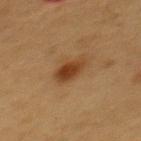illumination = cross-polarized illumination
imaging modality = ~15 mm tile from a whole-body skin photo
diameter = ~3.5 mm (longest diameter)
body site = the back
subject = male, aged approximately 55